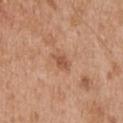Imaged during a routine full-body skin examination; the lesion was not biopsied and no histopathology is available. A male patient, approximately 55 years of age. A 15 mm close-up tile from a total-body photography series done for melanoma screening. Approximately 2.5 mm at its widest. From the chest.